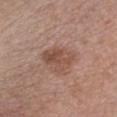Assessment:
Recorded during total-body skin imaging; not selected for excision or biopsy.
Clinical summary:
Cropped from a whole-body photographic skin survey; the tile spans about 15 mm. A female subject, roughly 30 years of age. The tile uses white-light illumination. The lesion is located on the chest.